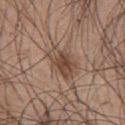* workup · total-body-photography surveillance lesion; no biopsy
* TBP lesion metrics · a classifier nevus-likeness of about 80/100
* image source · ~15 mm crop, total-body skin-cancer survey
* illumination · white-light illumination
* body site · the chest
* patient · male, aged 43 to 47